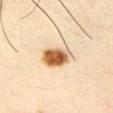* biopsy status · no biopsy performed (imaged during a skin exam)
* patient · male, about 40 years old
* lesion size · ~4.5 mm (longest diameter)
* illumination · cross-polarized
* location · the left upper arm
* imaging modality · ~15 mm crop, total-body skin-cancer survey